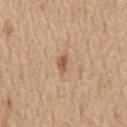Impression:
Captured during whole-body skin photography for melanoma surveillance; the lesion was not biopsied.
Context:
The patient is a male about 70 years old. The total-body-photography lesion software estimated an area of roughly 3 mm², a shape eccentricity near 0.9, and two-axis asymmetry of about 0.35. The software also gave a normalized border contrast of about 7. And it measured a border-irregularity rating of about 3.5/10, a within-lesion color-variation index near 1/10, and radial color variation of about 0.5. Measured at roughly 3 mm in maximum diameter. Located on the chest. A 15 mm close-up extracted from a 3D total-body photography capture. This is a white-light tile.